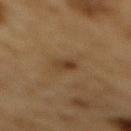Clinical impression:
The lesion was photographed on a routine skin check and not biopsied; there is no pathology result.
Acquisition and patient details:
The lesion's longest dimension is about 2.5 mm. This is a cross-polarized tile. The total-body-photography lesion software estimated a lesion area of about 3.5 mm², an outline eccentricity of about 0.8 (0 = round, 1 = elongated), and a symmetry-axis asymmetry near 0.2. And it measured a lesion–skin lightness drop of about 7 and a normalized border contrast of about 7. A male subject aged approximately 85 or older. A lesion tile, about 15 mm wide, cut from a 3D total-body photograph. The lesion is on the mid back.